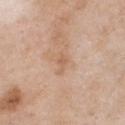Q: Is there a histopathology result?
A: imaged on a skin check; not biopsied
Q: Automated lesion metrics?
A: an area of roughly 3 mm², a shape eccentricity near 0.9, and two-axis asymmetry of about 0.35; a mean CIELAB color near L≈61 a*≈19 b*≈32 and a normalized border contrast of about 5.5; peripheral color asymmetry of about 0; a lesion-detection confidence of about 100/100
Q: How was the tile lit?
A: white-light illumination
Q: Lesion location?
A: the chest
Q: Patient demographics?
A: female, aged around 40
Q: Lesion size?
A: ~2.5 mm (longest diameter)
Q: How was this image acquired?
A: ~15 mm crop, total-body skin-cancer survey